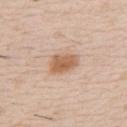Clinical impression: The lesion was photographed on a routine skin check and not biopsied; there is no pathology result. Context: From the chest. The subject is a male approximately 50 years of age. A lesion tile, about 15 mm wide, cut from a 3D total-body photograph.